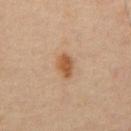Imaged during a routine full-body skin examination; the lesion was not biopsied and no histopathology is available. The lesion is on the chest. The lesion's longest dimension is about 3 mm. Automated image analysis of the tile measured a footprint of about 5 mm² and a shape eccentricity near 0.8. The software also gave an average lesion color of about L≈51 a*≈20 b*≈35 (CIELAB) and a normalized border contrast of about 9. The analysis additionally found a border-irregularity index near 2/10 and peripheral color asymmetry of about 0.5. And it measured a nevus-likeness score of about 95/100 and a detector confidence of about 100 out of 100 that the crop contains a lesion. Captured under cross-polarized illumination. A male patient aged approximately 45. A 15 mm crop from a total-body photograph taken for skin-cancer surveillance.